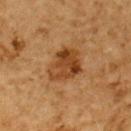No biopsy was performed on this lesion — it was imaged during a full skin examination and was not determined to be concerning.
A region of skin cropped from a whole-body photographic capture, roughly 15 mm wide.
A male patient, aged approximately 85 or older.
From the upper back.
The lesion-visualizer software estimated a footprint of about 12 mm², an eccentricity of roughly 0.6, and two-axis asymmetry of about 0.25. The analysis additionally found an average lesion color of about L≈37 a*≈21 b*≈34 (CIELAB), roughly 10 lightness units darker than nearby skin, and a lesion-to-skin contrast of about 8.5 (normalized; higher = more distinct). It also reported border irregularity of about 2.5 on a 0–10 scale, a within-lesion color-variation index near 5.5/10, and peripheral color asymmetry of about 2. The analysis additionally found an automated nevus-likeness rating near 85 out of 100 and a lesion-detection confidence of about 100/100.
The tile uses cross-polarized illumination.